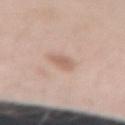patient: female, in their 20s
image source: 15 mm crop, total-body photography
anatomic site: the left forearm